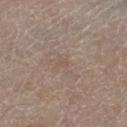Assessment:
The lesion was tiled from a total-body skin photograph and was not biopsied.
Context:
Measured at roughly 1.5 mm in maximum diameter. Captured under white-light illumination. The lesion is on the left thigh. A male patient, aged approximately 80. This image is a 15 mm lesion crop taken from a total-body photograph.